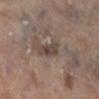Impression:
Recorded during total-body skin imaging; not selected for excision or biopsy.
Image and clinical context:
A female subject in their mid- to late 80s or older. The tile uses white-light illumination. The lesion is on the right lower leg. Automated tile analysis of the lesion measured a border-irregularity index near 3/10, a within-lesion color-variation index near 4/10, and a peripheral color-asymmetry measure near 1. And it measured a nevus-likeness score of about 0/100 and lesion-presence confidence of about 65/100. A 15 mm crop from a total-body photograph taken for skin-cancer surveillance. Measured at roughly 3.5 mm in maximum diameter.